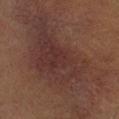Automated image analysis of the tile measured an average lesion color of about L≈27 a*≈15 b*≈18 (CIELAB) and about 5 CIELAB-L* units darker than the surrounding skin. The recorded lesion diameter is about 17.5 mm. Cropped from a total-body skin-imaging series; the visible field is about 15 mm. A male subject, in their 40s. Captured under cross-polarized illumination. The lesion is on the leg.